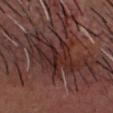{
  "biopsy_status": "not biopsied; imaged during a skin examination",
  "lesion_size": {
    "long_diameter_mm_approx": 10.0
  },
  "patient": {
    "sex": "male",
    "age_approx": 45
  },
  "automated_metrics": {
    "nevus_likeness_0_100": 0,
    "lesion_detection_confidence_0_100": 15
  },
  "site": "head or neck",
  "image": {
    "source": "total-body photography crop",
    "field_of_view_mm": 15
  }
}